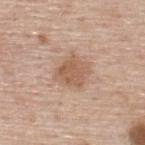* biopsy status — no biopsy performed (imaged during a skin exam)
* patient — male, aged around 75
* anatomic site — the upper back
* size — ≈3.5 mm
* tile lighting — white-light
* acquisition — ~15 mm crop, total-body skin-cancer survey